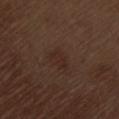workup: catalogued during a skin exam; not biopsied
illumination: white-light illumination
patient: male, approximately 70 years of age
acquisition: 15 mm crop, total-body photography
location: the left thigh
lesion size: about 3 mm
automated lesion analysis: a lesion area of about 5.5 mm², an eccentricity of roughly 0.75, and two-axis asymmetry of about 0.3; about 4 CIELAB-L* units darker than the surrounding skin and a normalized lesion–skin contrast near 4.5; a border-irregularity rating of about 2.5/10 and internal color variation of about 2.5 on a 0–10 scale